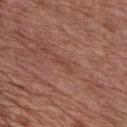The patient is a male in their 70s.
The total-body-photography lesion software estimated an area of roughly 2.5 mm², an outline eccentricity of about 0.9 (0 = round, 1 = elongated), and a shape-asymmetry score of about 0.4 (0 = symmetric). And it measured a lesion color around L≈45 a*≈23 b*≈26 in CIELAB and about 6 CIELAB-L* units darker than the surrounding skin. The software also gave a border-irregularity index near 4/10 and peripheral color asymmetry of about 0.
The recorded lesion diameter is about 3 mm.
Located on the chest.
Captured under white-light illumination.
A 15 mm close-up extracted from a 3D total-body photography capture.